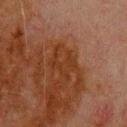Impression: Recorded during total-body skin imaging; not selected for excision or biopsy. Acquisition and patient details: A male subject, aged around 80. Measured at roughly 3.5 mm in maximum diameter. The lesion is located on the upper back. This image is a 15 mm lesion crop taken from a total-body photograph. Imaged with cross-polarized lighting.